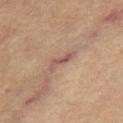notes: total-body-photography surveillance lesion; no biopsy | lesion diameter: ~3.5 mm (longest diameter) | tile lighting: cross-polarized | image: ~15 mm crop, total-body skin-cancer survey | subject: female, aged around 65 | body site: the left leg | automated metrics: a lesion area of about 4 mm², a shape eccentricity near 0.9, and a symmetry-axis asymmetry near 0.4; a lesion–skin lightness drop of about 9 and a lesion-to-skin contrast of about 7 (normalized; higher = more distinct).